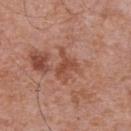The lesion was tiled from a total-body skin photograph and was not biopsied.
Cropped from a total-body skin-imaging series; the visible field is about 15 mm.
This is a white-light tile.
A male patient in their 70s.
Located on the chest.
About 3.5 mm across.